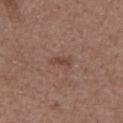Clinical impression:
No biopsy was performed on this lesion — it was imaged during a full skin examination and was not determined to be concerning.